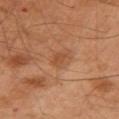patient: male, approximately 50 years of age
image source: total-body-photography crop, ~15 mm field of view
anatomic site: the left arm
automated lesion analysis: a footprint of about 3.5 mm² and two-axis asymmetry of about 0.4; an automated nevus-likeness rating near 10 out of 100